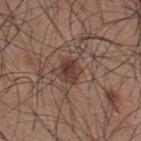Captured during whole-body skin photography for melanoma surveillance; the lesion was not biopsied. About 3 mm across. A male subject approximately 35 years of age. Captured under white-light illumination. On the upper back. A 15 mm crop from a total-body photograph taken for skin-cancer surveillance.